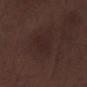Assessment: Part of a total-body skin-imaging series; this lesion was reviewed on a skin check and was not flagged for biopsy. Context: The subject is a male roughly 70 years of age. On the left lower leg. About 3.5 mm across. A 15 mm crop from a total-body photograph taken for skin-cancer surveillance. An algorithmic analysis of the crop reported an eccentricity of roughly 0.8. Captured under white-light illumination.